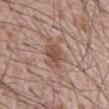Image and clinical context: About 5 mm across. Cropped from a total-body skin-imaging series; the visible field is about 15 mm. Located on the abdomen. A male subject aged approximately 70. Imaged with white-light lighting.